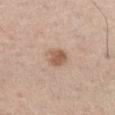The lesion was photographed on a routine skin check and not biopsied; there is no pathology result. The lesion-visualizer software estimated a lesion area of about 5.5 mm², an outline eccentricity of about 0.5 (0 = round, 1 = elongated), and a symmetry-axis asymmetry near 0.2. And it measured about 11 CIELAB-L* units darker than the surrounding skin. And it measured a border-irregularity index near 2/10, a within-lesion color-variation index near 2.5/10, and a peripheral color-asymmetry measure near 1. Located on the left thigh. A 15 mm crop from a total-body photograph taken for skin-cancer surveillance. About 2.5 mm across. The subject is a male about 75 years old. This is a white-light tile.